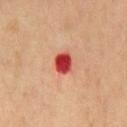| field | value |
|---|---|
| notes | total-body-photography surveillance lesion; no biopsy |
| automated metrics | an area of roughly 5 mm² and a symmetry-axis asymmetry near 0.15; border irregularity of about 1.5 on a 0–10 scale, a within-lesion color-variation index near 3/10, and peripheral color asymmetry of about 1 |
| lighting | cross-polarized illumination |
| acquisition | total-body-photography crop, ~15 mm field of view |
| anatomic site | the chest |
| subject | male, in their mid- to late 60s |